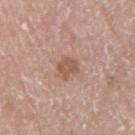biopsy status = no biopsy performed (imaged during a skin exam); image = ~15 mm crop, total-body skin-cancer survey; patient = male, roughly 70 years of age; illumination = white-light; site = the right upper arm.